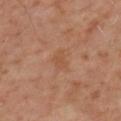<case>
<biopsy_status>not biopsied; imaged during a skin examination</biopsy_status>
<site>mid back</site>
<patient>
  <sex>male</sex>
  <age_approx>60</age_approx>
</patient>
<lesion_size>
  <long_diameter_mm_approx>2.5</long_diameter_mm_approx>
</lesion_size>
<image>
  <source>total-body photography crop</source>
  <field_of_view_mm>15</field_of_view_mm>
</image>
<lighting>cross-polarized</lighting>
</case>